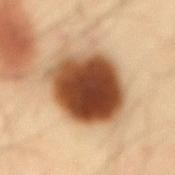workup: imaged on a skin check; not biopsied.